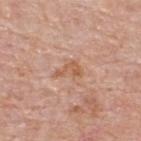A male patient aged around 75. The lesion's longest dimension is about 3 mm. A 15 mm close-up tile from a total-body photography series done for melanoma screening. Automated image analysis of the tile measured an outline eccentricity of about 0.85 (0 = round, 1 = elongated). The analysis additionally found a border-irregularity index near 6/10 and a within-lesion color-variation index near 2.5/10. On the upper back. Captured under white-light illumination.